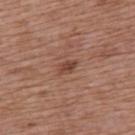anatomic site: the upper back
lighting: white-light illumination
image: ~15 mm tile from a whole-body skin photo
automated metrics: an average lesion color of about L≈44 a*≈22 b*≈28 (CIELAB), roughly 9 lightness units darker than nearby skin, and a normalized lesion–skin contrast near 7.5
patient: female, aged 73–77